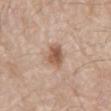Part of a total-body skin-imaging series; this lesion was reviewed on a skin check and was not flagged for biopsy.
The lesion-visualizer software estimated a shape-asymmetry score of about 0.25 (0 = symmetric).
From the mid back.
The subject is a male aged 78 to 82.
This is a white-light tile.
Cropped from a total-body skin-imaging series; the visible field is about 15 mm.